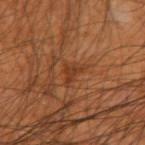<lesion>
<biopsy_status>not biopsied; imaged during a skin examination</biopsy_status>
<site>left forearm</site>
<image>
  <source>total-body photography crop</source>
  <field_of_view_mm>15</field_of_view_mm>
</image>
<patient>
  <sex>male</sex>
  <age_approx>45</age_approx>
</patient>
</lesion>